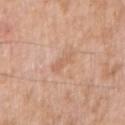biopsy status: no biopsy performed (imaged during a skin exam)
lighting: white-light illumination
site: the right upper arm
diameter: about 2.5 mm
patient: male, about 55 years old
imaging modality: total-body-photography crop, ~15 mm field of view